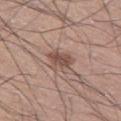Impression: The lesion was photographed on a routine skin check and not biopsied; there is no pathology result. Acquisition and patient details: A male subject in their mid-70s. A roughly 15 mm field-of-view crop from a total-body skin photograph. The lesion is located on the left thigh. The lesion-visualizer software estimated a normalized border contrast of about 7.5. The software also gave a border-irregularity index near 3.5/10 and peripheral color asymmetry of about 1. The tile uses white-light illumination.